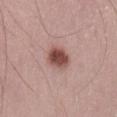The lesion was tiled from a total-body skin photograph and was not biopsied. Automated tile analysis of the lesion measured a mean CIELAB color near L≈49 a*≈23 b*≈24 and about 15 CIELAB-L* units darker than the surrounding skin. The lesion is located on the leg. The lesion's longest dimension is about 3.5 mm. A roughly 15 mm field-of-view crop from a total-body skin photograph. A male subject aged 53 to 57. Captured under white-light illumination.